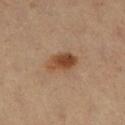notes: no biopsy performed (imaged during a skin exam)
imaging modality: total-body-photography crop, ~15 mm field of view
patient: female, in their 40s
tile lighting: cross-polarized illumination
location: the right lower leg
automated lesion analysis: a shape eccentricity near 0.8; a lesion–skin lightness drop of about 12 and a normalized border contrast of about 9.5; a border-irregularity index near 2/10, a within-lesion color-variation index near 6/10, and a peripheral color-asymmetry measure near 2.5